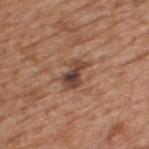notes — imaged on a skin check; not biopsied | body site — the upper back | image-analysis metrics — an average lesion color of about L≈43 a*≈20 b*≈26 (CIELAB), a lesion–skin lightness drop of about 12, and a lesion-to-skin contrast of about 10 (normalized; higher = more distinct) | image — ~15 mm crop, total-body skin-cancer survey | illumination — white-light | patient — male, aged approximately 65 | size — ~3.5 mm (longest diameter).